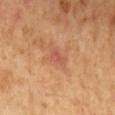| key | value |
|---|---|
| workup | imaged on a skin check; not biopsied |
| image source | total-body-photography crop, ~15 mm field of view |
| anatomic site | the mid back |
| patient | male, aged 58 to 62 |